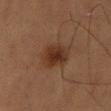Q: Was a biopsy performed?
A: imaged on a skin check; not biopsied
Q: What is the anatomic site?
A: the left thigh
Q: Who is the patient?
A: male, in their mid- to late 50s
Q: What is the imaging modality?
A: total-body-photography crop, ~15 mm field of view